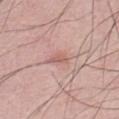This lesion was catalogued during total-body skin photography and was not selected for biopsy.
The lesion-visualizer software estimated an area of roughly 3 mm², a shape eccentricity near 0.85, and a shape-asymmetry score of about 0.25 (0 = symmetric). The analysis additionally found a lesion–skin lightness drop of about 8. And it measured border irregularity of about 2.5 on a 0–10 scale, a color-variation rating of about 1/10, and radial color variation of about 0. The software also gave a classifier nevus-likeness of about 0/100 and lesion-presence confidence of about 100/100.
Captured under white-light illumination.
On the chest.
A male patient aged 28–32.
A region of skin cropped from a whole-body photographic capture, roughly 15 mm wide.